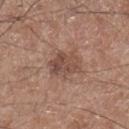No biopsy was performed on this lesion — it was imaged during a full skin examination and was not determined to be concerning. The lesion is located on the leg. The tile uses white-light illumination. Measured at roughly 4 mm in maximum diameter. Cropped from a total-body skin-imaging series; the visible field is about 15 mm. The patient is a male aged 58–62.